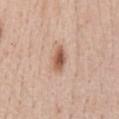Q: Is there a histopathology result?
A: total-body-photography surveillance lesion; no biopsy
Q: Where on the body is the lesion?
A: the front of the torso
Q: What kind of image is this?
A: ~15 mm crop, total-body skin-cancer survey
Q: Patient demographics?
A: male, roughly 60 years of age